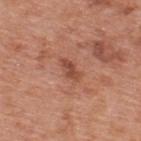Imaged during a routine full-body skin examination; the lesion was not biopsied and no histopathology is available. A close-up tile cropped from a whole-body skin photograph, about 15 mm across. The patient is a male roughly 70 years of age. Longest diameter approximately 3 mm. Captured under white-light illumination. Located on the upper back.